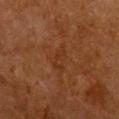Findings:
– imaging modality — ~15 mm tile from a whole-body skin photo
– subject — female, approximately 50 years of age
– body site — the chest